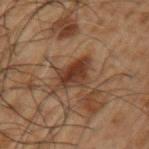The lesion was photographed on a routine skin check and not biopsied; there is no pathology result. On the left upper arm. A male patient about 50 years old. A region of skin cropped from a whole-body photographic capture, roughly 15 mm wide. Longest diameter approximately 5.5 mm. Automated tile analysis of the lesion measured a lesion area of about 9.5 mm² and a shape eccentricity near 0.85. The software also gave a nevus-likeness score of about 25/100. The tile uses cross-polarized illumination.